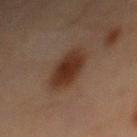workup = no biopsy performed (imaged during a skin exam)
diameter = about 5.5 mm
acquisition = 15 mm crop, total-body photography
subject = male, aged around 70
lighting = cross-polarized
body site = the abdomen